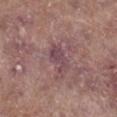notes = no biopsy performed (imaged during a skin exam) | anatomic site = the right lower leg | lighting = white-light illumination | imaging modality = ~15 mm tile from a whole-body skin photo | subject = female, approximately 75 years of age | lesion size = about 4 mm | automated lesion analysis = border irregularity of about 3.5 on a 0–10 scale, a color-variation rating of about 2.5/10, and radial color variation of about 1; a nevus-likeness score of about 0/100 and a lesion-detection confidence of about 95/100.